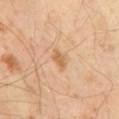The lesion's longest dimension is about 2.5 mm.
A male subject aged 63–67.
A roughly 15 mm field-of-view crop from a total-body skin photograph.